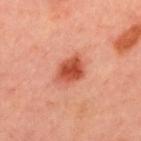Recorded during total-body skin imaging; not selected for excision or biopsy. The tile uses cross-polarized illumination. A 15 mm close-up extracted from a 3D total-body photography capture. A male patient, aged 48–52. Located on the upper back.